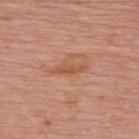notes: catalogued during a skin exam; not biopsied
subject: female, aged around 65
imaging modality: ~15 mm tile from a whole-body skin photo
lighting: white-light
size: ~3.5 mm (longest diameter)
anatomic site: the upper back
TBP lesion metrics: roughly 8 lightness units darker than nearby skin and a lesion-to-skin contrast of about 6 (normalized; higher = more distinct); a border-irregularity rating of about 4/10, a within-lesion color-variation index near 0.5/10, and peripheral color asymmetry of about 0; a classifier nevus-likeness of about 0/100 and a detector confidence of about 100 out of 100 that the crop contains a lesion